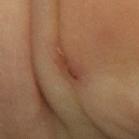The lesion was tiled from a total-body skin photograph and was not biopsied.
A roughly 15 mm field-of-view crop from a total-body skin photograph.
The subject is aged 58–62.
On the left forearm.
Imaged with cross-polarized lighting.
The recorded lesion diameter is about 3.5 mm.